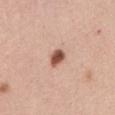<case>
  <biopsy_status>not biopsied; imaged during a skin examination</biopsy_status>
  <automated_metrics>
    <area_mm2_approx>4.5</area_mm2_approx>
    <eccentricity>0.75</eccentricity>
    <shape_asymmetry>0.2</shape_asymmetry>
    <border_irregularity_0_10>1.5</border_irregularity_0_10>
    <color_variation_0_10>3.5</color_variation_0_10>
    <peripheral_color_asymmetry>1.0</peripheral_color_asymmetry>
    <nevus_likeness_0_100>100</nevus_likeness_0_100>
    <lesion_detection_confidence_0_100>100</lesion_detection_confidence_0_100>
  </automated_metrics>
  <site>abdomen</site>
  <lighting>white-light</lighting>
  <lesion_size>
    <long_diameter_mm_approx>2.5</long_diameter_mm_approx>
  </lesion_size>
  <image>
    <source>total-body photography crop</source>
    <field_of_view_mm>15</field_of_view_mm>
  </image>
  <patient>
    <sex>female</sex>
    <age_approx>35</age_approx>
  </patient>
</case>